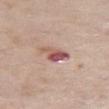{"biopsy_status": "not biopsied; imaged during a skin examination", "image": {"source": "total-body photography crop", "field_of_view_mm": 15}, "lesion_size": {"long_diameter_mm_approx": 3.5}, "patient": {"sex": "male", "age_approx": 60}, "automated_metrics": {"area_mm2_approx": 5.5, "eccentricity": 0.8, "shape_asymmetry": 0.4, "vs_skin_darker_L": 14.0, "vs_skin_contrast_norm": 9.0, "border_irregularity_0_10": 4.0, "color_variation_0_10": 10.0, "peripheral_color_asymmetry": 3.0}, "site": "left thigh", "lighting": "white-light"}